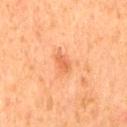Impression: This lesion was catalogued during total-body skin photography and was not selected for biopsy. Image and clinical context: The lesion is located on the mid back. A close-up tile cropped from a whole-body skin photograph, about 15 mm across. A male patient aged around 65.